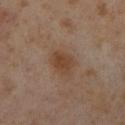<tbp_lesion>
<patient>
  <sex>female</sex>
  <age_approx>55</age_approx>
</patient>
<site>right lower leg</site>
<image>
  <source>total-body photography crop</source>
  <field_of_view_mm>15</field_of_view_mm>
</image>
</tbp_lesion>